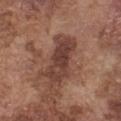Assessment: No biopsy was performed on this lesion — it was imaged during a full skin examination and was not determined to be concerning. Clinical summary: Imaged with white-light lighting. Approximately 6.5 mm at its widest. The lesion is on the front of the torso. A 15 mm close-up tile from a total-body photography series done for melanoma screening. Automated image analysis of the tile measured a symmetry-axis asymmetry near 0.25. The analysis additionally found a lesion color around L≈40 a*≈20 b*≈24 in CIELAB and a normalized lesion–skin contrast near 9.5. The software also gave a classifier nevus-likeness of about 0/100 and a lesion-detection confidence of about 100/100. A male patient, aged 73–77.